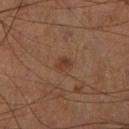No biopsy was performed on this lesion — it was imaged during a full skin examination and was not determined to be concerning.
The lesion's longest dimension is about 2 mm.
A male subject aged 63–67.
A region of skin cropped from a whole-body photographic capture, roughly 15 mm wide.
Imaged with cross-polarized lighting.
From the leg.